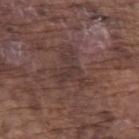Impression: The lesion was photographed on a routine skin check and not biopsied; there is no pathology result. Background: This is a white-light tile. Cropped from a total-body skin-imaging series; the visible field is about 15 mm. The subject is a male aged approximately 75. The lesion is on the left upper arm.